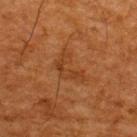workup = no biopsy performed (imaged during a skin exam) | image source = ~15 mm tile from a whole-body skin photo | lesion diameter = ≈4 mm | site = the upper back | automated lesion analysis = an area of roughly 5.5 mm², an eccentricity of roughly 0.8, and two-axis asymmetry of about 0.65; border irregularity of about 8.5 on a 0–10 scale, a within-lesion color-variation index near 0.5/10, and peripheral color asymmetry of about 0 | patient = male, aged 63–67 | illumination = cross-polarized.